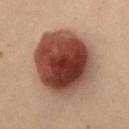Clinical impression: Imaged during a routine full-body skin examination; the lesion was not biopsied and no histopathology is available. Background: About 8.5 mm across. The lesion is located on the mid back. A 15 mm close-up extracted from a 3D total-body photography capture. A female subject, aged around 30. Imaged with cross-polarized lighting.